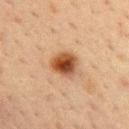A female patient, in their mid-50s.
The lesion is located on the chest.
Cropped from a whole-body photographic skin survey; the tile spans about 15 mm.